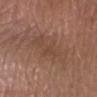The lesion was photographed on a routine skin check and not biopsied; there is no pathology result.
Automated image analysis of the tile measured a mean CIELAB color near L≈45 a*≈18 b*≈27.
A 15 mm crop from a total-body photograph taken for skin-cancer surveillance.
The patient is a male about 55 years old.
This is a white-light tile.
The lesion is on the right forearm.
Measured at roughly 6.5 mm in maximum diameter.